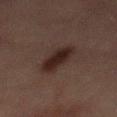Clinical impression:
Recorded during total-body skin imaging; not selected for excision or biopsy.
Background:
From the front of the torso. Captured under cross-polarized illumination. The total-body-photography lesion software estimated a lesion–skin lightness drop of about 8 and a normalized lesion–skin contrast near 10.5. The software also gave a nevus-likeness score of about 90/100 and a detector confidence of about 100 out of 100 that the crop contains a lesion. The recorded lesion diameter is about 5.5 mm. The subject is a male in their mid- to late 60s. A 15 mm crop from a total-body photograph taken for skin-cancer surveillance.